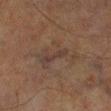| key | value |
|---|---|
| notes | total-body-photography surveillance lesion; no biopsy |
| lighting | cross-polarized |
| patient | male, in their mid-60s |
| acquisition | ~15 mm tile from a whole-body skin photo |
| lesion size | ≈6 mm |
| automated metrics | an area of roughly 12 mm² and a symmetry-axis asymmetry near 0.55; a lesion color around L≈30 a*≈12 b*≈19 in CIELAB and a normalized lesion–skin contrast near 4.5; a nevus-likeness score of about 0/100 |
| body site | the right lower leg |